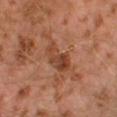  biopsy_status: not biopsied; imaged during a skin examination
  image:
    source: total-body photography crop
    field_of_view_mm: 15
  site: left lower leg
  patient:
    sex: male
    age_approx: 30
  lesion_size:
    long_diameter_mm_approx: 4.5
  lighting: cross-polarized
  automated_metrics:
    eccentricity: 0.9
    shape_asymmetry: 0.35
    nevus_likeness_0_100: 0